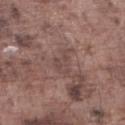Impression: Part of a total-body skin-imaging series; this lesion was reviewed on a skin check and was not flagged for biopsy. Acquisition and patient details: The subject is a male aged 73–77. Cropped from a whole-body photographic skin survey; the tile spans about 15 mm. Captured under white-light illumination. The total-body-photography lesion software estimated an average lesion color of about L≈45 a*≈18 b*≈20 (CIELAB), about 6 CIELAB-L* units darker than the surrounding skin, and a normalized lesion–skin contrast near 5. The lesion is located on the left lower leg. The lesion's longest dimension is about 3.5 mm.